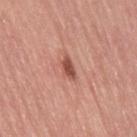{"biopsy_status": "not biopsied; imaged during a skin examination", "lighting": "white-light", "lesion_size": {"long_diameter_mm_approx": 3.0}, "patient": {"sex": "female", "age_approx": 65}, "automated_metrics": {"area_mm2_approx": 3.5, "eccentricity": 0.85, "shape_asymmetry": 0.3, "cielab_L": 52, "cielab_a": 27, "cielab_b": 28, "vs_skin_darker_L": 13.0, "lesion_detection_confidence_0_100": 100}, "image": {"source": "total-body photography crop", "field_of_view_mm": 15}, "site": "right thigh"}